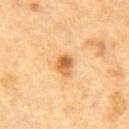biopsy_status: not biopsied; imaged during a skin examination
lighting: cross-polarized
patient:
  sex: male
  age_approx: 85+
image:
  source: total-body photography crop
  field_of_view_mm: 15
site: front of the torso